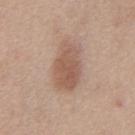{"biopsy_status": "not biopsied; imaged during a skin examination"}